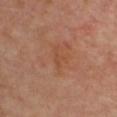Clinical impression: The lesion was photographed on a routine skin check and not biopsied; there is no pathology result. Background: Cropped from a total-body skin-imaging series; the visible field is about 15 mm. The lesion is located on the chest. The subject is a female aged around 60. This is a cross-polarized tile. Longest diameter approximately 2.5 mm.